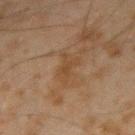Q: What is the anatomic site?
A: the right forearm
Q: Who is the patient?
A: male, approximately 45 years of age
Q: What kind of image is this?
A: 15 mm crop, total-body photography
Q: How was the tile lit?
A: cross-polarized illumination